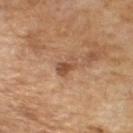Part of a total-body skin-imaging series; this lesion was reviewed on a skin check and was not flagged for biopsy. About 2.5 mm across. A male patient aged around 65. Cropped from a whole-body photographic skin survey; the tile spans about 15 mm. Automated tile analysis of the lesion measured a mean CIELAB color near L≈48 a*≈21 b*≈31 and about 10 CIELAB-L* units darker than the surrounding skin. It also reported a border-irregularity index near 3.5/10 and a within-lesion color-variation index near 2.5/10. The software also gave an automated nevus-likeness rating near 0 out of 100 and a lesion-detection confidence of about 100/100. Captured under cross-polarized illumination.